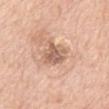Clinical impression: The lesion was tiled from a total-body skin photograph and was not biopsied. Background: A female patient, aged around 70. A lesion tile, about 15 mm wide, cut from a 3D total-body photograph. The lesion is on the mid back.